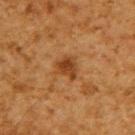notes: imaged on a skin check; not biopsied
imaging modality: total-body-photography crop, ~15 mm field of view
lighting: cross-polarized illumination
site: the upper back
lesion size: about 3 mm
subject: male, aged 58–62